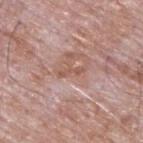- biopsy status: total-body-photography surveillance lesion; no biopsy
- lesion diameter: ≈3 mm
- subject: male, aged 63 to 67
- location: the upper back
- illumination: white-light illumination
- acquisition: 15 mm crop, total-body photography